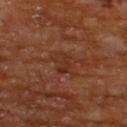Imaged during a routine full-body skin examination; the lesion was not biopsied and no histopathology is available. Located on the upper back. The subject is a male aged 63–67. The lesion-visualizer software estimated a border-irregularity rating of about 3.5/10 and a peripheral color-asymmetry measure near 0.5. The tile uses cross-polarized illumination. Approximately 2.5 mm at its widest. A region of skin cropped from a whole-body photographic capture, roughly 15 mm wide.